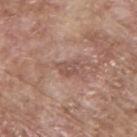biopsy status = no biopsy performed (imaged during a skin exam); patient = male, aged around 65; image = ~15 mm tile from a whole-body skin photo; lighting = white-light illumination; site = the upper back; diameter = ≈2.5 mm.